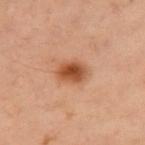Impression:
The lesion was photographed on a routine skin check and not biopsied; there is no pathology result.
Background:
About 3.5 mm across. Cropped from a whole-body photographic skin survey; the tile spans about 15 mm. The lesion is located on the left arm. Captured under cross-polarized illumination. The subject is a female aged around 55.